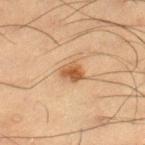The lesion was photographed on a routine skin check and not biopsied; there is no pathology result.
A male subject, in their mid-30s.
A region of skin cropped from a whole-body photographic capture, roughly 15 mm wide.
Located on the leg.